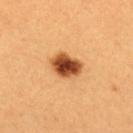{
  "biopsy_status": "not biopsied; imaged during a skin examination",
  "site": "back",
  "lighting": "cross-polarized",
  "automated_metrics": {
    "cielab_L": 49,
    "cielab_a": 27,
    "cielab_b": 41,
    "vs_skin_darker_L": 21.0
  },
  "patient": {
    "sex": "female",
    "age_approx": 25
  },
  "image": {
    "source": "total-body photography crop",
    "field_of_view_mm": 15
  }
}